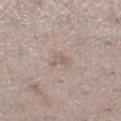Clinical impression: Captured during whole-body skin photography for melanoma surveillance; the lesion was not biopsied. Image and clinical context: Captured under white-light illumination. A 15 mm close-up extracted from a 3D total-body photography capture. From the left lower leg. The total-body-photography lesion software estimated an eccentricity of roughly 0.8 and two-axis asymmetry of about 0.5. And it measured a mean CIELAB color near L≈59 a*≈13 b*≈23, about 6 CIELAB-L* units darker than the surrounding skin, and a normalized lesion–skin contrast near 4.5. The analysis additionally found a border-irregularity rating of about 5/10 and internal color variation of about 1.5 on a 0–10 scale. And it measured a detector confidence of about 85 out of 100 that the crop contains a lesion. A female subject aged 38 to 42.